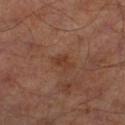image-analysis metrics: a lesion area of about 3 mm², a shape eccentricity near 0.8, and a shape-asymmetry score of about 0.4 (0 = symmetric)
lighting: cross-polarized illumination
anatomic site: the right lower leg
patient: male, roughly 65 years of age
diameter: about 2.5 mm
acquisition: total-body-photography crop, ~15 mm field of view